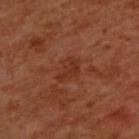Q: What kind of image is this?
A: ~15 mm tile from a whole-body skin photo
Q: Illumination type?
A: cross-polarized illumination
Q: Where on the body is the lesion?
A: the upper back
Q: Automated lesion metrics?
A: a footprint of about 5 mm², an eccentricity of roughly 0.8, and a symmetry-axis asymmetry near 0.35; a lesion color around L≈33 a*≈26 b*≈31 in CIELAB and a lesion–skin lightness drop of about 6; border irregularity of about 3.5 on a 0–10 scale, a within-lesion color-variation index near 2.5/10, and a peripheral color-asymmetry measure near 1; a nevus-likeness score of about 0/100 and a detector confidence of about 100 out of 100 that the crop contains a lesion
Q: How large is the lesion?
A: about 3 mm
Q: Patient demographics?
A: male, approximately 50 years of age